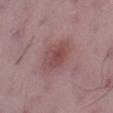Q: How large is the lesion?
A: ~4.5 mm (longest diameter)
Q: Patient demographics?
A: male, about 50 years old
Q: What is the anatomic site?
A: the right thigh
Q: What kind of image is this?
A: ~15 mm crop, total-body skin-cancer survey
Q: Illumination type?
A: white-light